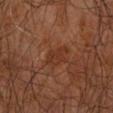Recorded during total-body skin imaging; not selected for excision or biopsy.
The lesion-visualizer software estimated an eccentricity of roughly 0.85.
A male patient aged around 65.
The tile uses cross-polarized illumination.
From the right upper arm.
A close-up tile cropped from a whole-body skin photograph, about 15 mm across.
Measured at roughly 4 mm in maximum diameter.